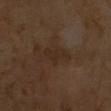Assessment:
No biopsy was performed on this lesion — it was imaged during a full skin examination and was not determined to be concerning.
Context:
A 15 mm close-up tile from a total-body photography series done for melanoma screening. The tile uses cross-polarized illumination. A male patient approximately 65 years of age. The lesion's longest dimension is about 3.5 mm.